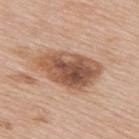Clinical impression:
Captured during whole-body skin photography for melanoma surveillance; the lesion was not biopsied.
Acquisition and patient details:
Approximately 7.5 mm at its widest. The lesion is on the upper back. The tile uses white-light illumination. The subject is a male about 80 years old. A lesion tile, about 15 mm wide, cut from a 3D total-body photograph.